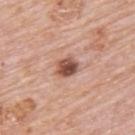  biopsy_status: not biopsied; imaged during a skin examination
  automated_metrics:
    cielab_L: 51
    cielab_a: 24
    cielab_b: 28
    vs_skin_contrast_norm: 11.0
  lesion_size:
    long_diameter_mm_approx: 3.0
  image:
    source: total-body photography crop
    field_of_view_mm: 15
  patient:
    sex: male
    age_approx: 80
  lighting: white-light
  site: upper back